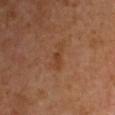Impression:
Captured during whole-body skin photography for melanoma surveillance; the lesion was not biopsied.
Clinical summary:
Longest diameter approximately 3.5 mm. The lesion is located on the left upper arm. A male patient approximately 60 years of age. This is a cross-polarized tile. A roughly 15 mm field-of-view crop from a total-body skin photograph.